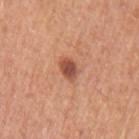<record>
<biopsy_status>not biopsied; imaged during a skin examination</biopsy_status>
<patient>
  <sex>male</sex>
  <age_approx>55</age_approx>
</patient>
<lighting>white-light</lighting>
<site>left upper arm</site>
<image>
  <source>total-body photography crop</source>
  <field_of_view_mm>15</field_of_view_mm>
</image>
<lesion_size>
  <long_diameter_mm_approx>2.5</long_diameter_mm_approx>
</lesion_size>
</record>